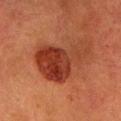Context:
Located on the head or neck. Cropped from a total-body skin-imaging series; the visible field is about 15 mm. A female patient about 40 years old.